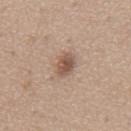Measured at roughly 3 mm in maximum diameter. The patient is a male in their mid- to late 60s. The lesion is located on the mid back. Cropped from a whole-body photographic skin survey; the tile spans about 15 mm.